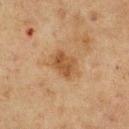The lesion was photographed on a routine skin check and not biopsied; there is no pathology result. A male patient roughly 75 years of age. From the chest. Imaged with cross-polarized lighting. A 15 mm close-up tile from a total-body photography series done for melanoma screening. About 4 mm across. Automated tile analysis of the lesion measured an average lesion color of about L≈42 a*≈17 b*≈31 (CIELAB), a lesion–skin lightness drop of about 8, and a lesion-to-skin contrast of about 7 (normalized; higher = more distinct). The software also gave a border-irregularity rating of about 2/10, internal color variation of about 3.5 on a 0–10 scale, and peripheral color asymmetry of about 1. The analysis additionally found a lesion-detection confidence of about 100/100.